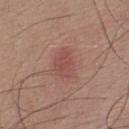Notes:
• biopsy status — catalogued during a skin exam; not biopsied
• body site — the chest
• lesion diameter — ~3.5 mm (longest diameter)
• tile lighting — white-light illumination
• subject — male, approximately 25 years of age
• acquisition — ~15 mm tile from a whole-body skin photo